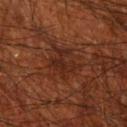No biopsy was performed on this lesion — it was imaged during a full skin examination and was not determined to be concerning.
The lesion is located on the right upper arm.
A male subject about 70 years old.
A close-up tile cropped from a whole-body skin photograph, about 15 mm across.